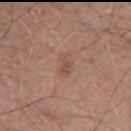Assessment: Captured during whole-body skin photography for melanoma surveillance; the lesion was not biopsied. Background: The patient is a male aged 48 to 52. The lesion is located on the chest. A region of skin cropped from a whole-body photographic capture, roughly 15 mm wide. The lesion's longest dimension is about 2.5 mm. Captured under white-light illumination.